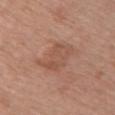{
  "biopsy_status": "not biopsied; imaged during a skin examination",
  "automated_metrics": {
    "cielab_L": 52,
    "cielab_a": 21,
    "cielab_b": 29,
    "vs_skin_darker_L": 7.0,
    "vs_skin_contrast_norm": 5.0,
    "lesion_detection_confidence_0_100": 100
  },
  "patient": {
    "sex": "female",
    "age_approx": 65
  },
  "lesion_size": {
    "long_diameter_mm_approx": 4.5
  },
  "site": "front of the torso",
  "image": {
    "source": "total-body photography crop",
    "field_of_view_mm": 15
  },
  "lighting": "white-light"
}